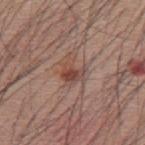Assessment: This lesion was catalogued during total-body skin photography and was not selected for biopsy. Acquisition and patient details: The lesion's longest dimension is about 3.5 mm. Cropped from a whole-body photographic skin survey; the tile spans about 15 mm. Captured under white-light illumination. A male patient, approximately 60 years of age. From the mid back.